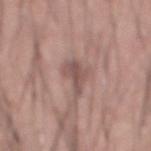Q: What kind of image is this?
A: ~15 mm crop, total-body skin-cancer survey
Q: Who is the patient?
A: male, roughly 60 years of age
Q: What did automated image analysis measure?
A: internal color variation of about 3 on a 0–10 scale and radial color variation of about 1; a nevus-likeness score of about 0/100 and a detector confidence of about 70 out of 100 that the crop contains a lesion
Q: What is the anatomic site?
A: the abdomen
Q: Lesion size?
A: about 4.5 mm